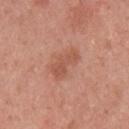{
  "biopsy_status": "not biopsied; imaged during a skin examination",
  "lighting": "white-light",
  "automated_metrics": {
    "cielab_L": 54,
    "cielab_a": 25,
    "cielab_b": 30,
    "vs_skin_darker_L": 8.0,
    "vs_skin_contrast_norm": 5.5,
    "nevus_likeness_0_100": 10,
    "lesion_detection_confidence_0_100": 100
  },
  "lesion_size": {
    "long_diameter_mm_approx": 4.0
  },
  "patient": {
    "sex": "male",
    "age_approx": 25
  },
  "image": {
    "source": "total-body photography crop",
    "field_of_view_mm": 15
  },
  "site": "upper back"
}